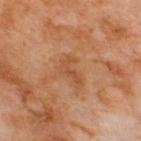  biopsy_status: not biopsied; imaged during a skin examination
  patient:
    sex: male
    age_approx: 70
  image:
    source: total-body photography crop
    field_of_view_mm: 15
  lesion_size:
    long_diameter_mm_approx: 3.5
  site: upper back
  lighting: cross-polarized